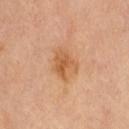Impression: Imaged during a routine full-body skin examination; the lesion was not biopsied and no histopathology is available. Clinical summary: The tile uses cross-polarized illumination. A close-up tile cropped from a whole-body skin photograph, about 15 mm across. The recorded lesion diameter is about 3.5 mm. A female patient, aged 58 to 62. Located on the abdomen.